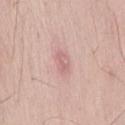workup: catalogued during a skin exam; not biopsied
lesion diameter: about 3 mm
image source: ~15 mm crop, total-body skin-cancer survey
site: the lower back
subject: male, aged 48 to 52
lighting: white-light